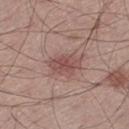<case>
  <biopsy_status>not biopsied; imaged during a skin examination</biopsy_status>
  <lesion_size>
    <long_diameter_mm_approx>4.5</long_diameter_mm_approx>
  </lesion_size>
  <image>
    <source>total-body photography crop</source>
    <field_of_view_mm>15</field_of_view_mm>
  </image>
  <automated_metrics>
    <area_mm2_approx>9.5</area_mm2_approx>
    <shape_asymmetry>0.2</shape_asymmetry>
  </automated_metrics>
  <patient>
    <sex>male</sex>
    <age_approx>55</age_approx>
  </patient>
  <site>left thigh</site>
  <lighting>white-light</lighting>
</case>